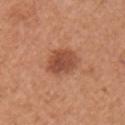Assessment: Recorded during total-body skin imaging; not selected for excision or biopsy. Background: This is a white-light tile. A close-up tile cropped from a whole-body skin photograph, about 15 mm across. Located on the right upper arm. A female subject aged approximately 50. The recorded lesion diameter is about 4 mm.